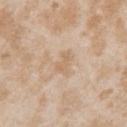Impression: Imaged during a routine full-body skin examination; the lesion was not biopsied and no histopathology is available. Image and clinical context: A 15 mm close-up tile from a total-body photography series done for melanoma screening. The lesion is on the right upper arm. A female subject, roughly 25 years of age.